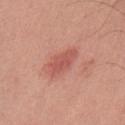Case summary:
– patient · male, aged approximately 45
– acquisition · 15 mm crop, total-body photography
– lesion size · ~5 mm (longest diameter)
– lighting · white-light illumination
– body site · the upper back
– automated lesion analysis · a border-irregularity rating of about 3/10 and a peripheral color-asymmetry measure near 0.5; an automated nevus-likeness rating near 95 out of 100 and lesion-presence confidence of about 100/100